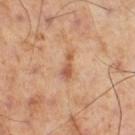biopsy status: catalogued during a skin exam; not biopsied.